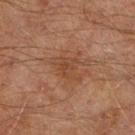| feature | finding |
|---|---|
| notes | catalogued during a skin exam; not biopsied |
| subject | male, about 65 years old |
| illumination | cross-polarized illumination |
| automated lesion analysis | a within-lesion color-variation index near 2.5/10 and a peripheral color-asymmetry measure near 1; a classifier nevus-likeness of about 0/100 and lesion-presence confidence of about 100/100 |
| site | the leg |
| acquisition | ~15 mm crop, total-body skin-cancer survey |